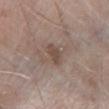biopsy status: imaged on a skin check; not biopsied | patient: female, in their mid-70s | lesion size: ~3 mm (longest diameter) | illumination: white-light illumination | site: the left lower leg | imaging modality: total-body-photography crop, ~15 mm field of view | automated metrics: a mean CIELAB color near L≈47 a*≈15 b*≈23 and a normalized border contrast of about 6.5; a classifier nevus-likeness of about 0/100 and lesion-presence confidence of about 100/100.